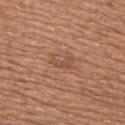Q: Was this lesion biopsied?
A: catalogued during a skin exam; not biopsied
Q: Automated lesion metrics?
A: a footprint of about 4 mm², an eccentricity of roughly 0.85, and two-axis asymmetry of about 0.45
Q: What kind of image is this?
A: ~15 mm tile from a whole-body skin photo
Q: What lighting was used for the tile?
A: white-light illumination
Q: Where on the body is the lesion?
A: the right upper arm
Q: How large is the lesion?
A: about 3 mm
Q: Patient demographics?
A: female, aged 53 to 57